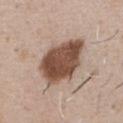biopsy_status: not biopsied; imaged during a skin examination
lesion_size:
  long_diameter_mm_approx: 6.5
automated_metrics:
  area_mm2_approx: 22.0
  eccentricity: 0.7
  shape_asymmetry: 0.2
  cielab_L: 49
  cielab_a: 18
  cielab_b: 27
  vs_skin_darker_L: 18.0
  border_irregularity_0_10: 2.5
patient:
  sex: male
  age_approx: 50
image:
  source: total-body photography crop
  field_of_view_mm: 15
lighting: white-light
site: chest